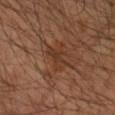{
  "biopsy_status": "not biopsied; imaged during a skin examination",
  "patient": {
    "age_approx": 55
  },
  "automated_metrics": {
    "area_mm2_approx": 5.5,
    "eccentricity": 0.75,
    "shape_asymmetry": 0.35,
    "vs_skin_darker_L": 6.0,
    "vs_skin_contrast_norm": 6.0
  },
  "lesion_size": {
    "long_diameter_mm_approx": 3.0
  },
  "image": {
    "source": "total-body photography crop",
    "field_of_view_mm": 15
  },
  "lighting": "cross-polarized",
  "site": "right forearm"
}